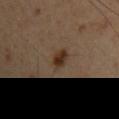Recorded during total-body skin imaging; not selected for excision or biopsy. On the left upper arm. A 15 mm crop from a total-body photograph taken for skin-cancer surveillance. The patient is a male in their 50s.